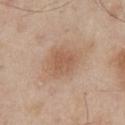Findings:
- workup: catalogued during a skin exam; not biopsied
- image-analysis metrics: a shape eccentricity near 0.55 and a symmetry-axis asymmetry near 0.25; a mean CIELAB color near L≈57 a*≈19 b*≈31, roughly 8 lightness units darker than nearby skin, and a normalized lesion–skin contrast near 5.5; a border-irregularity index near 2.5/10 and peripheral color asymmetry of about 1
- lighting: white-light
- body site: the chest
- subject: male, aged around 65
- size: ≈3.5 mm
- imaging modality: 15 mm crop, total-body photography